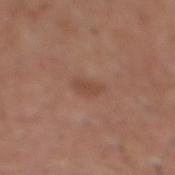{
  "biopsy_status": "not biopsied; imaged during a skin examination",
  "site": "mid back",
  "image": {
    "source": "total-body photography crop",
    "field_of_view_mm": 15
  },
  "lesion_size": {
    "long_diameter_mm_approx": 3.0
  },
  "patient": {
    "sex": "male",
    "age_approx": 75
  },
  "lighting": "white-light"
}